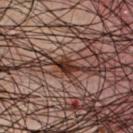<tbp_lesion>
<biopsy_status>not biopsied; imaged during a skin examination</biopsy_status>
<lighting>cross-polarized</lighting>
<lesion_size>
  <long_diameter_mm_approx>3.0</long_diameter_mm_approx>
</lesion_size>
<site>chest</site>
<patient>
  <sex>male</sex>
  <age_approx>45</age_approx>
</patient>
<image>
  <source>total-body photography crop</source>
  <field_of_view_mm>15</field_of_view_mm>
</image>
</tbp_lesion>